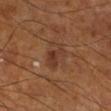biopsy_status: not biopsied; imaged during a skin examination
site: right lower leg
patient:
  sex: male
  age_approx: 70
image:
  source: total-body photography crop
  field_of_view_mm: 15